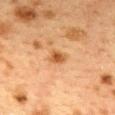Q: Was a biopsy performed?
A: no biopsy performed (imaged during a skin exam)
Q: Lesion size?
A: ~2.5 mm (longest diameter)
Q: What did automated image analysis measure?
A: roughly 10 lightness units darker than nearby skin and a normalized lesion–skin contrast near 8; an automated nevus-likeness rating near 45 out of 100 and lesion-presence confidence of about 100/100
Q: What are the patient's age and sex?
A: female, aged 38 to 42
Q: Where on the body is the lesion?
A: the back
Q: What lighting was used for the tile?
A: cross-polarized illumination
Q: What kind of image is this?
A: ~15 mm tile from a whole-body skin photo